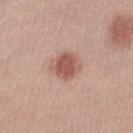follow-up=imaged on a skin check; not biopsied | site=the left lower leg | patient=female, aged 18 to 22 | image source=~15 mm crop, total-body skin-cancer survey.